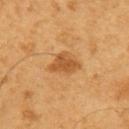Captured during whole-body skin photography for melanoma surveillance; the lesion was not biopsied. The recorded lesion diameter is about 4 mm. A male subject, roughly 60 years of age. A close-up tile cropped from a whole-body skin photograph, about 15 mm across. The tile uses cross-polarized illumination. Located on the right upper arm.